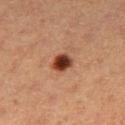Findings:
- notes · catalogued during a skin exam; not biopsied
- image · 15 mm crop, total-body photography
- lesion diameter · about 2.5 mm
- illumination · cross-polarized
- body site · the right thigh
- subject · female, about 40 years old